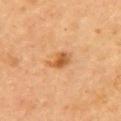Recorded during total-body skin imaging; not selected for excision or biopsy. The lesion is on the upper back. A lesion tile, about 15 mm wide, cut from a 3D total-body photograph. A female subject, aged around 60. The tile uses cross-polarized illumination.